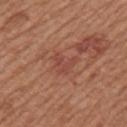Context:
Located on the left upper arm. A male patient, roughly 55 years of age. A close-up tile cropped from a whole-body skin photograph, about 15 mm across.